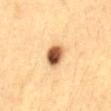Clinical impression:
This lesion was catalogued during total-body skin photography and was not selected for biopsy.
Acquisition and patient details:
The lesion is located on the front of the torso. Captured under cross-polarized illumination. Automated image analysis of the tile measured a border-irregularity rating of about 1.5/10, a color-variation rating of about 9.5/10, and radial color variation of about 3.5. The subject is a female roughly 30 years of age. Longest diameter approximately 3 mm. This image is a 15 mm lesion crop taken from a total-body photograph.